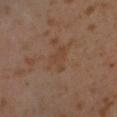Assessment: The lesion was tiled from a total-body skin photograph and was not biopsied. Context: Automated tile analysis of the lesion measured a footprint of about 4.5 mm², a shape eccentricity near 0.8, and a shape-asymmetry score of about 0.5 (0 = symmetric). The software also gave internal color variation of about 1 on a 0–10 scale and a peripheral color-asymmetry measure near 0.5. And it measured a classifier nevus-likeness of about 0/100 and a detector confidence of about 100 out of 100 that the crop contains a lesion. A region of skin cropped from a whole-body photographic capture, roughly 15 mm wide. About 3.5 mm across. Located on the left lower leg. A male patient, roughly 30 years of age.